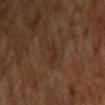illumination — cross-polarized illumination; patient — male, in their mid-60s; size — ~3 mm (longest diameter); acquisition — ~15 mm crop, total-body skin-cancer survey.